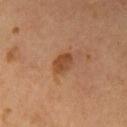The lesion was photographed on a routine skin check and not biopsied; there is no pathology result.